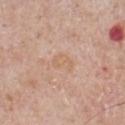The lesion was tiled from a total-body skin photograph and was not biopsied.
A male subject, about 65 years old.
Measured at roughly 2.5 mm in maximum diameter.
Captured under white-light illumination.
From the chest.
The lesion-visualizer software estimated an area of roughly 3.5 mm², a shape eccentricity near 0.8, and two-axis asymmetry of about 0.5. The analysis additionally found a border-irregularity rating of about 5/10, a color-variation rating of about 0.5/10, and radial color variation of about 0.
A 15 mm close-up extracted from a 3D total-body photography capture.